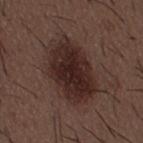Imaged during a routine full-body skin examination; the lesion was not biopsied and no histopathology is available. About 8 mm across. A roughly 15 mm field-of-view crop from a total-body skin photograph. The tile uses white-light illumination. The lesion is located on the mid back. The patient is a male aged 48–52.